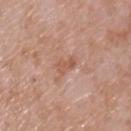biopsy_status: not biopsied; imaged during a skin examination
lighting: white-light
patient:
  sex: male
  age_approx: 50
site: chest
image:
  source: total-body photography crop
  field_of_view_mm: 15